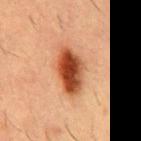Imaged during a routine full-body skin examination; the lesion was not biopsied and no histopathology is available.
A male patient approximately 55 years of age.
The lesion is located on the mid back.
This image is a 15 mm lesion crop taken from a total-body photograph.